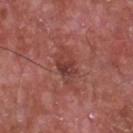biopsy status: no biopsy performed (imaged during a skin exam) | acquisition: ~15 mm crop, total-body skin-cancer survey | body site: the front of the torso | subject: male, about 65 years old.